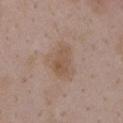Cropped from a total-body skin-imaging series; the visible field is about 15 mm. The lesion's longest dimension is about 4.5 mm. The lesion-visualizer software estimated a lesion color around L≈54 a*≈16 b*≈27 in CIELAB, about 7 CIELAB-L* units darker than the surrounding skin, and a normalized lesion–skin contrast near 6. A female subject, about 35 years old. The tile uses white-light illumination. The lesion is located on the chest.